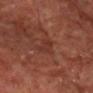Notes:
- follow-up — no biopsy performed (imaged during a skin exam)
- patient — male, in their mid-60s
- location — the head or neck
- acquisition — ~15 mm tile from a whole-body skin photo
- illumination — cross-polarized